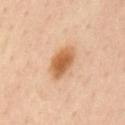A 15 mm close-up tile from a total-body photography series done for melanoma screening.
The recorded lesion diameter is about 4.5 mm.
This is a cross-polarized tile.
From the mid back.
A male subject aged 53–57.